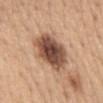Part of a total-body skin-imaging series; this lesion was reviewed on a skin check and was not flagged for biopsy. A lesion tile, about 15 mm wide, cut from a 3D total-body photograph. Measured at roughly 5.5 mm in maximum diameter. An algorithmic analysis of the crop reported a footprint of about 18 mm², an outline eccentricity of about 0.65 (0 = round, 1 = elongated), and a symmetry-axis asymmetry near 0.15. It also reported a lesion color around L≈50 a*≈20 b*≈29 in CIELAB and a lesion–skin lightness drop of about 18. The analysis additionally found internal color variation of about 7 on a 0–10 scale and a peripheral color-asymmetry measure near 2. The lesion is located on the mid back. This is a white-light tile. A female patient, about 65 years old.